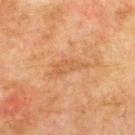The lesion was photographed on a routine skin check and not biopsied; there is no pathology result.
A 15 mm close-up tile from a total-body photography series done for melanoma screening.
A male patient, approximately 75 years of age.
On the upper back.
Approximately 4.5 mm at its widest.
The tile uses cross-polarized illumination.